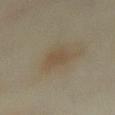| field | value |
|---|---|
| workup | catalogued during a skin exam; not biopsied |
| automated metrics | a footprint of about 5 mm² and a shape-asymmetry score of about 0.15 (0 = symmetric); a normalized border contrast of about 5; internal color variation of about 1.5 on a 0–10 scale and radial color variation of about 0.5; a classifier nevus-likeness of about 15/100 and a lesion-detection confidence of about 100/100 |
| lighting | cross-polarized |
| subject | female, aged approximately 35 |
| lesion diameter | ≈3.5 mm |
| image | total-body-photography crop, ~15 mm field of view |
| anatomic site | the abdomen |